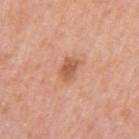Recorded during total-body skin imaging; not selected for excision or biopsy.
A female subject aged 38–42.
An algorithmic analysis of the crop reported an eccentricity of roughly 0.7 and a shape-asymmetry score of about 0.25 (0 = symmetric). The software also gave a mean CIELAB color near L≈58 a*≈25 b*≈34, about 11 CIELAB-L* units darker than the surrounding skin, and a normalized border contrast of about 7. It also reported a nevus-likeness score of about 60/100 and a lesion-detection confidence of about 100/100.
A roughly 15 mm field-of-view crop from a total-body skin photograph.
This is a white-light tile.
The lesion is located on the left upper arm.